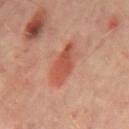Recorded during total-body skin imaging; not selected for excision or biopsy.
On the mid back.
Measured at roughly 5 mm in maximum diameter.
The tile uses cross-polarized illumination.
The subject is a male aged around 65.
A 15 mm crop from a total-body photograph taken for skin-cancer surveillance.
Automated tile analysis of the lesion measured a symmetry-axis asymmetry near 0.2. And it measured a color-variation rating of about 4.5/10 and a peripheral color-asymmetry measure near 1.5. It also reported lesion-presence confidence of about 100/100.